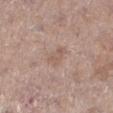notes: catalogued during a skin exam; not biopsied
size: about 2.5 mm
image source: ~15 mm tile from a whole-body skin photo
TBP lesion metrics: a shape eccentricity near 0.7 and two-axis asymmetry of about 0.4; an average lesion color of about L≈57 a*≈17 b*≈25 (CIELAB), about 6 CIELAB-L* units darker than the surrounding skin, and a lesion-to-skin contrast of about 5 (normalized; higher = more distinct); a border-irregularity index near 3.5/10, a color-variation rating of about 1.5/10, and a peripheral color-asymmetry measure near 0.5
lighting: white-light
site: the right lower leg
subject: female, about 40 years old